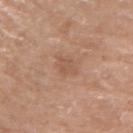Notes:
* workup — total-body-photography surveillance lesion; no biopsy
* patient — male, aged approximately 70
* automated metrics — an average lesion color of about L≈55 a*≈20 b*≈30 (CIELAB), roughly 6 lightness units darker than nearby skin, and a lesion-to-skin contrast of about 4.5 (normalized; higher = more distinct); lesion-presence confidence of about 100/100
* lesion size — about 2.5 mm
* body site — the left upper arm
* acquisition — 15 mm crop, total-body photography